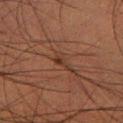biopsy_status: not biopsied; imaged during a skin examination
patient:
  sex: male
  age_approx: 50
lighting: cross-polarized
site: left lower leg
lesion_size:
  long_diameter_mm_approx: 2.5
image:
  source: total-body photography crop
  field_of_view_mm: 15